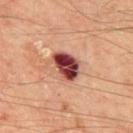Imaged during a routine full-body skin examination; the lesion was not biopsied and no histopathology is available. A male patient aged 63 to 67. This image is a 15 mm lesion crop taken from a total-body photograph. From the upper back.